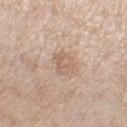Impression: No biopsy was performed on this lesion — it was imaged during a full skin examination and was not determined to be concerning. Acquisition and patient details: A female patient, approximately 55 years of age. A 15 mm close-up extracted from a 3D total-body photography capture. Approximately 3 mm at its widest. Located on the right lower leg.